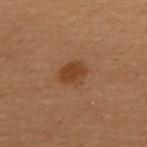This lesion was catalogued during total-body skin photography and was not selected for biopsy.
Approximately 3 mm at its widest.
A female patient, aged around 50.
The tile uses cross-polarized illumination.
Cropped from a total-body skin-imaging series; the visible field is about 15 mm.
On the upper back.